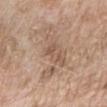Q: Is there a histopathology result?
A: total-body-photography surveillance lesion; no biopsy
Q: What kind of image is this?
A: 15 mm crop, total-body photography
Q: What is the anatomic site?
A: the right upper arm
Q: What is the lesion's diameter?
A: about 8 mm
Q: Illumination type?
A: white-light illumination
Q: Patient demographics?
A: female, aged around 75